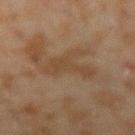- notes: catalogued during a skin exam; not biopsied
- subject: male, aged 43–47
- illumination: cross-polarized
- image-analysis metrics: a lesion area of about 7 mm², a shape eccentricity near 0.85, and a shape-asymmetry score of about 0.65 (0 = symmetric); an average lesion color of about L≈35 a*≈14 b*≈25 (CIELAB) and roughly 5 lightness units darker than nearby skin; a classifier nevus-likeness of about 0/100 and a lesion-detection confidence of about 100/100
- image source: 15 mm crop, total-body photography
- anatomic site: the right forearm
- diameter: ≈4.5 mm